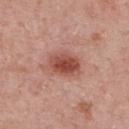notes: total-body-photography surveillance lesion; no biopsy | body site: the upper back | tile lighting: white-light | image: 15 mm crop, total-body photography | subject: male, aged around 65 | lesion diameter: about 4 mm.